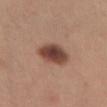notes: total-body-photography surveillance lesion; no biopsy
lesion size: ≈4.5 mm
acquisition: ~15 mm tile from a whole-body skin photo
patient: female, approximately 30 years of age
location: the abdomen
illumination: white-light illumination